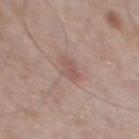Case summary:
- site — the chest
- patient — male, roughly 75 years of age
- lesion diameter — ≈2.5 mm
- image — total-body-photography crop, ~15 mm field of view
- automated lesion analysis — an area of roughly 4.5 mm², an outline eccentricity of about 0.6 (0 = round, 1 = elongated), and two-axis asymmetry of about 0.3; about 7 CIELAB-L* units darker than the surrounding skin and a normalized lesion–skin contrast near 5; an automated nevus-likeness rating near 0 out of 100 and lesion-presence confidence of about 100/100